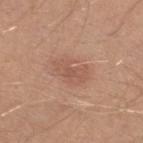No biopsy was performed on this lesion — it was imaged during a full skin examination and was not determined to be concerning. A roughly 15 mm field-of-view crop from a total-body skin photograph. A male subject, aged 28 to 32. Measured at roughly 4.5 mm in maximum diameter. Captured under white-light illumination. Located on the left lower leg.